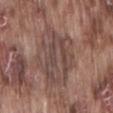workup = imaged on a skin check; not biopsied | image source = ~15 mm crop, total-body skin-cancer survey | site = the lower back | tile lighting = white-light | automated lesion analysis = a mean CIELAB color near L≈45 a*≈17 b*≈21; a within-lesion color-variation index near 5/10 and peripheral color asymmetry of about 2; a detector confidence of about 70 out of 100 that the crop contains a lesion | subject = male, aged 73–77.